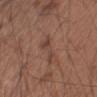No biopsy was performed on this lesion — it was imaged during a full skin examination and was not determined to be concerning.
An algorithmic analysis of the crop reported roughly 7 lightness units darker than nearby skin. The analysis additionally found border irregularity of about 7 on a 0–10 scale, a color-variation rating of about 1/10, and peripheral color asymmetry of about 0.5.
On the right upper arm.
A 15 mm close-up extracted from a 3D total-body photography capture.
Captured under white-light illumination.
The subject is a male about 55 years old.
The lesion's longest dimension is about 3.5 mm.